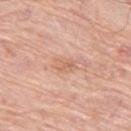Imaged during a routine full-body skin examination; the lesion was not biopsied and no histopathology is available.
A male subject aged approximately 80.
A roughly 15 mm field-of-view crop from a total-body skin photograph.
Approximately 2.5 mm at its widest.
On the left thigh.
This is a white-light tile.